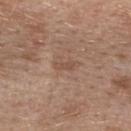Imaged during a routine full-body skin examination; the lesion was not biopsied and no histopathology is available. A female patient in their 40s. Automated tile analysis of the lesion measured an automated nevus-likeness rating near 0 out of 100 and a lesion-detection confidence of about 100/100. Longest diameter approximately 2.5 mm. The lesion is located on the upper back. Cropped from a whole-body photographic skin survey; the tile spans about 15 mm. The tile uses white-light illumination.